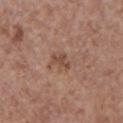follow-up = no biopsy performed (imaged during a skin exam)
acquisition = ~15 mm crop, total-body skin-cancer survey
size = about 2.5 mm
TBP lesion metrics = an eccentricity of roughly 0.8 and a symmetry-axis asymmetry near 0.5; a classifier nevus-likeness of about 0/100
site = the chest
subject = female, about 65 years old